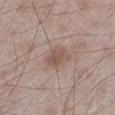Imaged during a routine full-body skin examination; the lesion was not biopsied and no histopathology is available.
Captured under white-light illumination.
Located on the left lower leg.
A roughly 15 mm field-of-view crop from a total-body skin photograph.
A male patient, aged approximately 60.
The recorded lesion diameter is about 3 mm.
Automated image analysis of the tile measured an average lesion color of about L≈52 a*≈18 b*≈24 (CIELAB), a lesion–skin lightness drop of about 9, and a lesion-to-skin contrast of about 6.5 (normalized; higher = more distinct). The analysis additionally found a border-irregularity index near 2.5/10, a color-variation rating of about 2/10, and a peripheral color-asymmetry measure near 0.5. The software also gave a classifier nevus-likeness of about 70/100 and lesion-presence confidence of about 100/100.